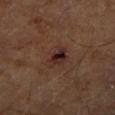The lesion was tiled from a total-body skin photograph and was not biopsied.
The tile uses cross-polarized illumination.
The patient is roughly 65 years of age.
A 15 mm crop from a total-body photograph taken for skin-cancer surveillance.
Longest diameter approximately 3 mm.
Located on the right lower leg.